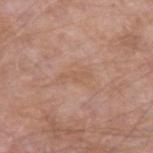Recorded during total-body skin imaging; not selected for excision or biopsy.
On the arm.
Captured under white-light illumination.
Cropped from a total-body skin-imaging series; the visible field is about 15 mm.
A male patient roughly 65 years of age.
Longest diameter approximately 3 mm.